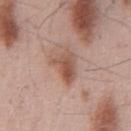Findings:
* site — the chest
* tile lighting — white-light
* patient — male, aged 53–57
* automated metrics — an average lesion color of about L≈54 a*≈20 b*≈28 (CIELAB) and a lesion–skin lightness drop of about 11; a border-irregularity rating of about 4.5/10, a within-lesion color-variation index near 5.5/10, and radial color variation of about 2; a classifier nevus-likeness of about 50/100 and a lesion-detection confidence of about 100/100
* imaging modality — ~15 mm tile from a whole-body skin photo